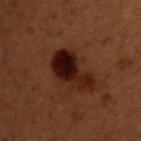Clinical impression:
The lesion was tiled from a total-body skin photograph and was not biopsied.
Clinical summary:
The subject is a male about 50 years old. A roughly 15 mm field-of-view crop from a total-body skin photograph. The lesion is on the upper back.